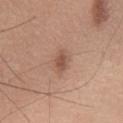No biopsy was performed on this lesion — it was imaged during a full skin examination and was not determined to be concerning. A 15 mm crop from a total-body photograph taken for skin-cancer surveillance. A male patient about 55 years old. The lesion is on the chest.